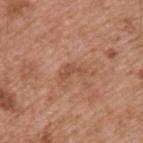follow-up = no biopsy performed (imaged during a skin exam) | acquisition = 15 mm crop, total-body photography | subject = male, aged 53 to 57 | TBP lesion metrics = a lesion color around L≈51 a*≈23 b*≈32 in CIELAB, about 7 CIELAB-L* units darker than the surrounding skin, and a normalized lesion–skin contrast near 5.5 | location = the back | illumination = white-light.